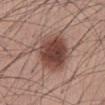A 15 mm close-up extracted from a 3D total-body photography capture. The patient is a male aged around 40. The lesion is on the abdomen. On biopsy, histopathology showed a dysplastic (Clark) nevus.